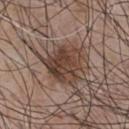Clinical impression: Part of a total-body skin-imaging series; this lesion was reviewed on a skin check and was not flagged for biopsy. Context: A male subject, approximately 55 years of age. A 15 mm close-up tile from a total-body photography series done for melanoma screening. The lesion is located on the chest.